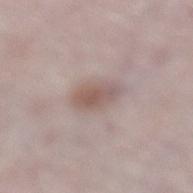follow-up = catalogued during a skin exam; not biopsied | image source = ~15 mm crop, total-body skin-cancer survey | lesion diameter = ≈4.5 mm | lighting = white-light illumination | location = the left lower leg | subject = female, about 50 years old.